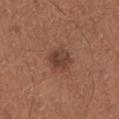workup=no biopsy performed (imaged during a skin exam)
automated lesion analysis=a mean CIELAB color near L≈40 a*≈21 b*≈26 and a normalized border contrast of about 8; a border-irregularity rating of about 2.5/10 and a within-lesion color-variation index near 4/10; a lesion-detection confidence of about 100/100
body site=the right lower leg
tile lighting=white-light
image source=15 mm crop, total-body photography
subject=male, aged 63 to 67
diameter=about 3.5 mm